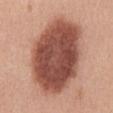Clinical impression: Part of a total-body skin-imaging series; this lesion was reviewed on a skin check and was not flagged for biopsy. Acquisition and patient details: Located on the abdomen. A female subject, aged approximately 40. The tile uses white-light illumination. A 15 mm close-up extracted from a 3D total-body photography capture. Automated image analysis of the tile measured about 19 CIELAB-L* units darker than the surrounding skin and a lesion-to-skin contrast of about 12 (normalized; higher = more distinct). And it measured a border-irregularity index near 1/10, a within-lesion color-variation index near 5.5/10, and a peripheral color-asymmetry measure near 1.5.